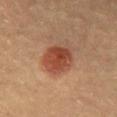No biopsy was performed on this lesion — it was imaged during a full skin examination and was not determined to be concerning.